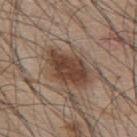The lesion was tiled from a total-body skin photograph and was not biopsied.
A 15 mm close-up tile from a total-body photography series done for melanoma screening.
An algorithmic analysis of the crop reported a shape eccentricity near 0.8. And it measured a mean CIELAB color near L≈41 a*≈18 b*≈26 and a normalized lesion–skin contrast near 10.5. The software also gave a color-variation rating of about 3/10. The analysis additionally found a classifier nevus-likeness of about 90/100 and lesion-presence confidence of about 100/100.
Longest diameter approximately 5.5 mm.
A male subject about 45 years old.
This is a white-light tile.
Located on the upper back.